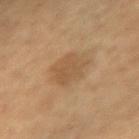No biopsy was performed on this lesion — it was imaged during a full skin examination and was not determined to be concerning.
Approximately 4 mm at its widest.
A 15 mm crop from a total-body photograph taken for skin-cancer surveillance.
Automated image analysis of the tile measured a footprint of about 9.5 mm², an eccentricity of roughly 0.45, and a shape-asymmetry score of about 0.2 (0 = symmetric).
A female subject aged 58 to 62.
The tile uses cross-polarized illumination.
The lesion is on the right forearm.